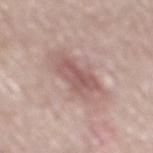Impression:
The lesion was photographed on a routine skin check and not biopsied; there is no pathology result.
Image and clinical context:
A roughly 15 mm field-of-view crop from a total-body skin photograph. The lesion's longest dimension is about 5.5 mm. A male patient, aged 78 to 82. The lesion is on the mid back. The lesion-visualizer software estimated an area of roughly 13 mm². It also reported border irregularity of about 4 on a 0–10 scale.